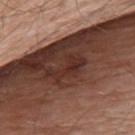{"biopsy_status": "not biopsied; imaged during a skin examination", "lesion_size": {"long_diameter_mm_approx": 5.0}, "site": "upper back", "patient": {"sex": "male", "age_approx": 80}, "automated_metrics": {"nevus_likeness_0_100": 0}, "lighting": "white-light", "image": {"source": "total-body photography crop", "field_of_view_mm": 15}}